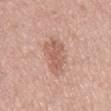| field | value |
|---|---|
| workup | total-body-photography surveillance lesion; no biopsy |
| patient | male, about 70 years old |
| location | the mid back |
| tile lighting | white-light |
| image | 15 mm crop, total-body photography |